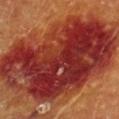Q: Automated lesion metrics?
A: about 15 CIELAB-L* units darker than the surrounding skin and a normalized border contrast of about 13; a within-lesion color-variation index near 10/10; a classifier nevus-likeness of about 0/100 and lesion-presence confidence of about 100/100
Q: What are the patient's age and sex?
A: female, about 80 years old
Q: What is the imaging modality?
A: total-body-photography crop, ~15 mm field of view
Q: What is the anatomic site?
A: the chest
Q: What is the lesion's diameter?
A: about 17.5 mm